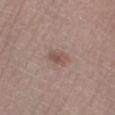This lesion was catalogued during total-body skin photography and was not selected for biopsy. The recorded lesion diameter is about 2.5 mm. The tile uses white-light illumination. On the right lower leg. A region of skin cropped from a whole-body photographic capture, roughly 15 mm wide. A female patient aged around 55.